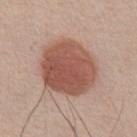The lesion was tiled from a total-body skin photograph and was not biopsied.
The lesion is located on the front of the torso.
Approximately 6.5 mm at its widest.
The subject is a male in their mid-50s.
Cropped from a whole-body photographic skin survey; the tile spans about 15 mm.
Captured under white-light illumination.